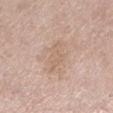Q: What is the anatomic site?
A: the left lower leg
Q: How was this image acquired?
A: ~15 mm crop, total-body skin-cancer survey
Q: Who is the patient?
A: female, roughly 55 years of age
Q: What lighting was used for the tile?
A: white-light illumination